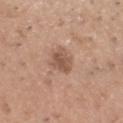The lesion was photographed on a routine skin check and not biopsied; there is no pathology result. Longest diameter approximately 3 mm. A male patient approximately 35 years of age. The lesion is located on the head or neck. Captured under white-light illumination. An algorithmic analysis of the crop reported a lesion area of about 6 mm² and an eccentricity of roughly 0.55. And it measured an average lesion color of about L≈54 a*≈19 b*≈28 (CIELAB), about 10 CIELAB-L* units darker than the surrounding skin, and a normalized border contrast of about 7. This image is a 15 mm lesion crop taken from a total-body photograph.